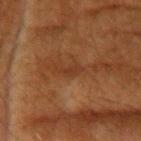Notes:
– workup · no biopsy performed (imaged during a skin exam)
– tile lighting · cross-polarized
– image-analysis metrics · a shape eccentricity near 0.9; a border-irregularity index near 3.5/10 and a color-variation rating of about 0/10; a classifier nevus-likeness of about 0/100
– patient · female, approximately 80 years of age
– lesion diameter · about 3 mm
– location · the head or neck
– image · total-body-photography crop, ~15 mm field of view